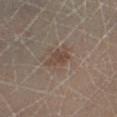<record>
  <biopsy_status>not biopsied; imaged during a skin examination</biopsy_status>
</record>